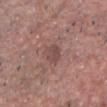– biopsy status: imaged on a skin check; not biopsied